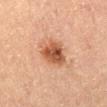follow-up: catalogued during a skin exam; not biopsied | acquisition: ~15 mm tile from a whole-body skin photo | anatomic site: the leg | subject: female, roughly 60 years of age.